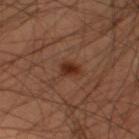<lesion>
<biopsy_status>not biopsied; imaged during a skin examination</biopsy_status>
<automated_metrics>
  <cielab_L>28</cielab_L>
  <cielab_a>22</cielab_a>
  <cielab_b>27</cielab_b>
  <vs_skin_darker_L>10.0</vs_skin_darker_L>
  <border_irregularity_0_10>2.0</border_irregularity_0_10>
  <color_variation_0_10>3.0</color_variation_0_10>
  <peripheral_color_asymmetry>1.0</peripheral_color_asymmetry>
  <nevus_likeness_0_100>95</nevus_likeness_0_100>
  <lesion_detection_confidence_0_100>100</lesion_detection_confidence_0_100>
</automated_metrics>
<image>
  <source>total-body photography crop</source>
  <field_of_view_mm>15</field_of_view_mm>
</image>
<site>leg</site>
<lighting>cross-polarized</lighting>
<patient>
  <sex>male</sex>
  <age_approx>45</age_approx>
</patient>
</lesion>